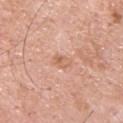A 15 mm crop from a total-body photograph taken for skin-cancer surveillance.
Approximately 2.5 mm at its widest.
The lesion is on the upper back.
This is a white-light tile.
The patient is a male roughly 60 years of age.